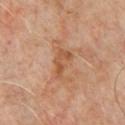Findings:
• patient: male, in their mid-60s
• location: the chest
• lighting: cross-polarized
• imaging modality: 15 mm crop, total-body photography
• lesion diameter: ~4 mm (longest diameter)
• TBP lesion metrics: border irregularity of about 4.5 on a 0–10 scale, internal color variation of about 3.5 on a 0–10 scale, and radial color variation of about 1